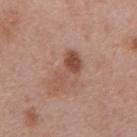The lesion was photographed on a routine skin check and not biopsied; there is no pathology result.
The recorded lesion diameter is about 5.5 mm.
A 15 mm close-up tile from a total-body photography series done for melanoma screening.
The tile uses white-light illumination.
The patient is a male approximately 70 years of age.
On the mid back.